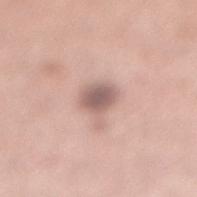No biopsy was performed on this lesion — it was imaged during a full skin examination and was not determined to be concerning. This is a white-light tile. A 15 mm close-up extracted from a 3D total-body photography capture. A female patient, aged approximately 30. Approximately 3 mm at its widest. From the right lower leg. An algorithmic analysis of the crop reported a shape eccentricity near 0.55 and two-axis asymmetry of about 0.15. The software also gave an average lesion color of about L≈58 a*≈18 b*≈21 (CIELAB), roughly 14 lightness units darker than nearby skin, and a normalized border contrast of about 9.